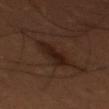The lesion was photographed on a routine skin check and not biopsied; there is no pathology result. Imaged with cross-polarized lighting. On the left thigh. The lesion-visualizer software estimated a shape eccentricity near 0.75 and two-axis asymmetry of about 0.2. It also reported an average lesion color of about L≈20 a*≈16 b*≈22 (CIELAB), a lesion–skin lightness drop of about 7, and a normalized lesion–skin contrast near 8. It also reported border irregularity of about 3 on a 0–10 scale and radial color variation of about 1. A 15 mm crop from a total-body photograph taken for skin-cancer surveillance. A male subject, aged 48 to 52.